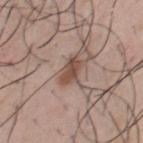The lesion is located on the chest.
Captured under white-light illumination.
The patient is a male about 30 years old.
A 15 mm close-up tile from a total-body photography series done for melanoma screening.
About 3 mm across.